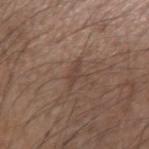Assessment:
No biopsy was performed on this lesion — it was imaged during a full skin examination and was not determined to be concerning.
Context:
An algorithmic analysis of the crop reported a shape eccentricity near 0.9. It also reported an average lesion color of about L≈42 a*≈16 b*≈24 (CIELAB) and a normalized lesion–skin contrast near 5.5. The analysis additionally found a nevus-likeness score of about 0/100. Cropped from a total-body skin-imaging series; the visible field is about 15 mm. Measured at roughly 3 mm in maximum diameter. The patient is a male roughly 55 years of age. The lesion is on the left forearm.